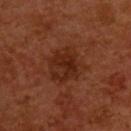No biopsy was performed on this lesion — it was imaged during a full skin examination and was not determined to be concerning. This is a cross-polarized tile. The lesion is located on the back. Approximately 4.5 mm at its widest. The patient is a male in their mid-50s. A 15 mm close-up tile from a total-body photography series done for melanoma screening.